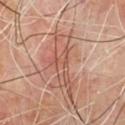| key | value |
|---|---|
| follow-up | catalogued during a skin exam; not biopsied |
| acquisition | ~15 mm tile from a whole-body skin photo |
| patient | male, aged around 65 |
| lesion size | ~7.5 mm (longest diameter) |
| anatomic site | the chest |
| lighting | cross-polarized illumination |